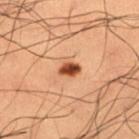Clinical impression: The lesion was tiled from a total-body skin photograph and was not biopsied. Image and clinical context: The patient is a male about 50 years old. A 15 mm crop from a total-body photograph taken for skin-cancer surveillance. This is a cross-polarized tile. From the left thigh.